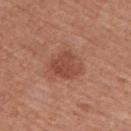Q: Was this lesion biopsied?
A: catalogued during a skin exam; not biopsied
Q: Where on the body is the lesion?
A: the upper back
Q: Lesion size?
A: ≈4 mm
Q: How was the tile lit?
A: white-light
Q: What kind of image is this?
A: 15 mm crop, total-body photography
Q: Automated lesion metrics?
A: an area of roughly 8.5 mm², a shape eccentricity near 0.6, and a shape-asymmetry score of about 0.25 (0 = symmetric); border irregularity of about 3 on a 0–10 scale and radial color variation of about 1; an automated nevus-likeness rating near 50 out of 100
Q: Who is the patient?
A: male, aged 53–57